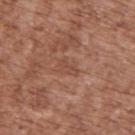Part of a total-body skin-imaging series; this lesion was reviewed on a skin check and was not flagged for biopsy. An algorithmic analysis of the crop reported a footprint of about 3.5 mm², an eccentricity of roughly 0.8, and a shape-asymmetry score of about 0.35 (0 = symmetric). It also reported an automated nevus-likeness rating near 0 out of 100 and a detector confidence of about 95 out of 100 that the crop contains a lesion. A male subject about 75 years old. A region of skin cropped from a whole-body photographic capture, roughly 15 mm wide. This is a white-light tile. Measured at roughly 2.5 mm in maximum diameter. Located on the upper back.